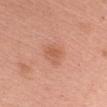notes — total-body-photography surveillance lesion; no biopsy
illumination — white-light illumination
body site — the left upper arm
image source — ~15 mm crop, total-body skin-cancer survey
subject — female, in their 50s
lesion diameter — ≈3 mm